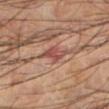No biopsy was performed on this lesion — it was imaged during a full skin examination and was not determined to be concerning. The lesion is on the left lower leg. A male patient in their 60s. Approximately 3.5 mm at its widest. A region of skin cropped from a whole-body photographic capture, roughly 15 mm wide. The tile uses cross-polarized illumination.